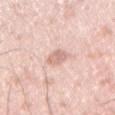Context: A lesion tile, about 15 mm wide, cut from a 3D total-body photograph. A male subject aged 33 to 37. Longest diameter approximately 2.5 mm. The lesion is located on the right upper arm.